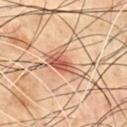Assessment: This lesion was catalogued during total-body skin photography and was not selected for biopsy. Image and clinical context: From the chest. The tile uses cross-polarized illumination. A male subject approximately 70 years of age. A 15 mm close-up extracted from a 3D total-body photography capture. Measured at roughly 5 mm in maximum diameter.